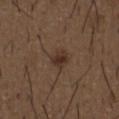<lesion>
<patient>
  <sex>male</sex>
  <age_approx>50</age_approx>
</patient>
<site>chest</site>
<image>
  <source>total-body photography crop</source>
  <field_of_view_mm>15</field_of_view_mm>
</image>
</lesion>